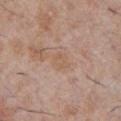Impression: Captured during whole-body skin photography for melanoma surveillance; the lesion was not biopsied. Context: From the chest. An algorithmic analysis of the crop reported a footprint of about 4 mm². It also reported a classifier nevus-likeness of about 0/100 and a detector confidence of about 100 out of 100 that the crop contains a lesion. A close-up tile cropped from a whole-body skin photograph, about 15 mm across. The patient is a male roughly 30 years of age. The lesion's longest dimension is about 2.5 mm.